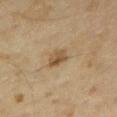Acquisition and patient details:
Measured at roughly 3 mm in maximum diameter. Captured under cross-polarized illumination. A male patient roughly 65 years of age. The lesion-visualizer software estimated a lesion area of about 4.5 mm², a shape eccentricity near 0.75, and two-axis asymmetry of about 0.2. The software also gave a lesion color around L≈50 a*≈14 b*≈34 in CIELAB and about 10 CIELAB-L* units darker than the surrounding skin. The software also gave a classifier nevus-likeness of about 50/100 and a detector confidence of about 100 out of 100 that the crop contains a lesion. This image is a 15 mm lesion crop taken from a total-body photograph.